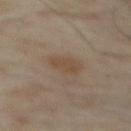| feature | finding |
|---|---|
| biopsy status | no biopsy performed (imaged during a skin exam) |
| imaging modality | ~15 mm crop, total-body skin-cancer survey |
| body site | the chest |
| patient | male, about 65 years old |
| lesion size | about 3 mm |
| automated lesion analysis | an eccentricity of roughly 0.6 and a symmetry-axis asymmetry near 0.2; a mean CIELAB color near L≈44 a*≈14 b*≈26, a lesion–skin lightness drop of about 6, and a lesion-to-skin contrast of about 6 (normalized; higher = more distinct); an automated nevus-likeness rating near 55 out of 100 and a detector confidence of about 100 out of 100 that the crop contains a lesion |
| lighting | cross-polarized illumination |